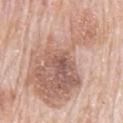biopsy status = catalogued during a skin exam; not biopsied
diameter = ≈13 mm
location = the mid back
subject = male, aged 78–82
imaging modality = total-body-photography crop, ~15 mm field of view
TBP lesion metrics = a shape eccentricity near 0.85 and a symmetry-axis asymmetry near 0.45; a mean CIELAB color near L≈62 a*≈19 b*≈27, about 11 CIELAB-L* units darker than the surrounding skin, and a normalized border contrast of about 7; a nevus-likeness score of about 0/100 and lesion-presence confidence of about 100/100
lighting = white-light illumination